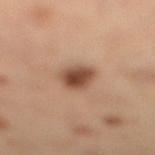A 15 mm close-up extracted from a 3D total-body photography capture. Longest diameter approximately 4 mm. The tile uses cross-polarized illumination. The lesion is located on the right lower leg. The total-body-photography lesion software estimated border irregularity of about 2 on a 0–10 scale, a color-variation rating of about 4.5/10, and radial color variation of about 1.5. The software also gave a nevus-likeness score of about 95/100 and a detector confidence of about 100 out of 100 that the crop contains a lesion. The subject is a female aged 53–57.